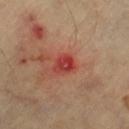Notes:
– follow-up — no biopsy performed (imaged during a skin exam)
– acquisition — 15 mm crop, total-body photography
– site — the right lower leg
– subject — aged 58 to 62